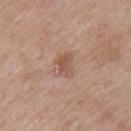Imaged during a routine full-body skin examination; the lesion was not biopsied and no histopathology is available. The lesion is on the arm. About 2.5 mm across. The total-body-photography lesion software estimated a lesion area of about 4.5 mm², a shape eccentricity near 0.75, and a shape-asymmetry score of about 0.4 (0 = symmetric). The software also gave a lesion color around L≈54 a*≈19 b*≈29 in CIELAB and a lesion–skin lightness drop of about 9. The analysis additionally found a classifier nevus-likeness of about 5/100 and a detector confidence of about 100 out of 100 that the crop contains a lesion. Cropped from a whole-body photographic skin survey; the tile spans about 15 mm. The patient is a male aged around 50.